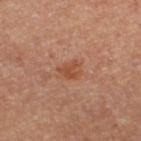The lesion was photographed on a routine skin check and not biopsied; there is no pathology result.
The patient is female.
Located on the left thigh.
A 15 mm close-up extracted from a 3D total-body photography capture.
The lesion's longest dimension is about 2.5 mm.